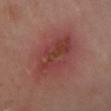subject — female, roughly 55 years of age | image — ~15 mm tile from a whole-body skin photo | lesion size — about 7 mm | anatomic site — the left lower leg | tile lighting — cross-polarized | TBP lesion metrics — an area of roughly 22 mm² and an outline eccentricity of about 0.8 (0 = round, 1 = elongated); a mean CIELAB color near L≈41 a*≈27 b*≈24, a lesion–skin lightness drop of about 8, and a normalized border contrast of about 6.5.